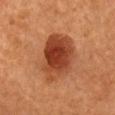Impression:
This lesion was catalogued during total-body skin photography and was not selected for biopsy.
Image and clinical context:
From the chest. Measured at roughly 6.5 mm in maximum diameter. Captured under cross-polarized illumination. Automated image analysis of the tile measured a footprint of about 22 mm² and a shape eccentricity near 0.7. It also reported roughly 13 lightness units darker than nearby skin. The software also gave border irregularity of about 2 on a 0–10 scale. A female subject, aged 63–67. A roughly 15 mm field-of-view crop from a total-body skin photograph.